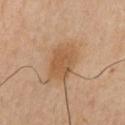Q: Was this lesion biopsied?
A: imaged on a skin check; not biopsied
Q: What is the anatomic site?
A: the left upper arm
Q: What is the lesion's diameter?
A: about 4.5 mm
Q: Who is the patient?
A: male, roughly 40 years of age
Q: How was the tile lit?
A: cross-polarized illumination
Q: Automated lesion metrics?
A: a footprint of about 10 mm², an eccentricity of roughly 0.8, and two-axis asymmetry of about 0.15; a mean CIELAB color near L≈53 a*≈19 b*≈35 and a lesion-to-skin contrast of about 7 (normalized; higher = more distinct)
Q: What is the imaging modality?
A: ~15 mm tile from a whole-body skin photo